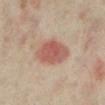<record>
  <biopsy_status>not biopsied; imaged during a skin examination</biopsy_status>
  <lighting>cross-polarized</lighting>
  <automated_metrics>
    <border_irregularity_0_10>2.0</border_irregularity_0_10>
    <color_variation_0_10>3.5</color_variation_0_10>
    <peripheral_color_asymmetry>1.0</peripheral_color_asymmetry>
    <nevus_likeness_0_100>100</nevus_likeness_0_100>
    <lesion_detection_confidence_0_100>100</lesion_detection_confidence_0_100>
  </automated_metrics>
  <image>
    <source>total-body photography crop</source>
    <field_of_view_mm>15</field_of_view_mm>
  </image>
  <lesion_size>
    <long_diameter_mm_approx>5.0</long_diameter_mm_approx>
  </lesion_size>
  <patient>
    <sex>female</sex>
    <age_approx>35</age_approx>
  </patient>
  <site>left lower leg</site>
</record>